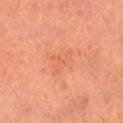Q: Was a biopsy performed?
A: catalogued during a skin exam; not biopsied
Q: Illumination type?
A: cross-polarized illumination
Q: What is the anatomic site?
A: the head or neck
Q: Automated lesion metrics?
A: a lesion color around L≈62 a*≈29 b*≈37 in CIELAB, a lesion–skin lightness drop of about 5, and a lesion-to-skin contrast of about 3 (normalized; higher = more distinct)
Q: Patient demographics?
A: female, aged 48 to 52
Q: What is the imaging modality?
A: total-body-photography crop, ~15 mm field of view
Q: What is the lesion's diameter?
A: ≈3.5 mm